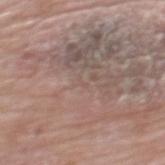The lesion was tiled from a total-body skin photograph and was not biopsied.
A region of skin cropped from a whole-body photographic capture, roughly 15 mm wide.
A female patient aged approximately 60.
This is a white-light tile.
The lesion is located on the upper back.